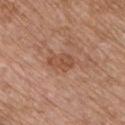The lesion was tiled from a total-body skin photograph and was not biopsied.
Cropped from a total-body skin-imaging series; the visible field is about 15 mm.
About 3.5 mm across.
This is a white-light tile.
Located on the chest.
The subject is a male about 65 years old.